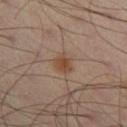Imaged during a routine full-body skin examination; the lesion was not biopsied and no histopathology is available.
A region of skin cropped from a whole-body photographic capture, roughly 15 mm wide.
A male patient aged 43–47.
The lesion-visualizer software estimated an outline eccentricity of about 0.3 (0 = round, 1 = elongated) and a shape-asymmetry score of about 0.25 (0 = symmetric). The software also gave a mean CIELAB color near L≈44 a*≈17 b*≈28, roughly 8 lightness units darker than nearby skin, and a normalized border contrast of about 7.5. The software also gave internal color variation of about 2.5 on a 0–10 scale.
The lesion is on the right lower leg.
Longest diameter approximately 2.5 mm.